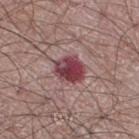Assessment: Recorded during total-body skin imaging; not selected for excision or biopsy. Background: A male subject approximately 65 years of age. On the right thigh. Cropped from a whole-body photographic skin survey; the tile spans about 15 mm. Imaged with white-light lighting. The recorded lesion diameter is about 3.5 mm.